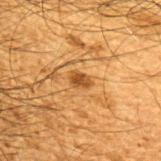Clinical summary:
The lesion is located on the upper back. A 15 mm close-up tile from a total-body photography series done for melanoma screening. A male patient roughly 65 years of age.